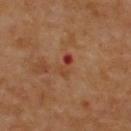Notes:
• workup — catalogued during a skin exam; not biopsied
• lighting — cross-polarized
• location — the back
• imaging modality — 15 mm crop, total-body photography
• patient — male, approximately 70 years of age
• lesion size — ≈3 mm